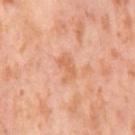{"biopsy_status": "not biopsied; imaged during a skin examination"}